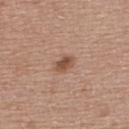Image and clinical context: From the upper back. A close-up tile cropped from a whole-body skin photograph, about 15 mm across. A female subject, aged 58 to 62. Longest diameter approximately 3 mm.